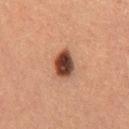<tbp_lesion>
  <biopsy_status>not biopsied; imaged during a skin examination</biopsy_status>
  <site>right thigh</site>
  <patient>
    <sex>female</sex>
    <age_approx>40</age_approx>
  </patient>
  <image>
    <source>total-body photography crop</source>
    <field_of_view_mm>15</field_of_view_mm>
  </image>
</tbp_lesion>